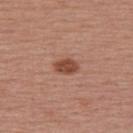Background:
The subject is a female aged approximately 55. The lesion is located on the upper back. A 15 mm close-up extracted from a 3D total-body photography capture.